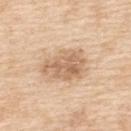Imaged during a routine full-body skin examination; the lesion was not biopsied and no histopathology is available.
This image is a 15 mm lesion crop taken from a total-body photograph.
The lesion is located on the upper back.
A female patient aged around 55.
The tile uses white-light illumination.